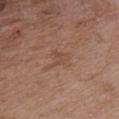Recorded during total-body skin imaging; not selected for excision or biopsy.
A male patient aged around 50.
The tile uses white-light illumination.
A 15 mm close-up extracted from a 3D total-body photography capture.
The lesion is located on the chest.